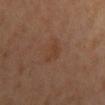Recorded during total-body skin imaging; not selected for excision or biopsy. A region of skin cropped from a whole-body photographic capture, roughly 15 mm wide. The lesion is on the back. The patient is a female aged 48 to 52.